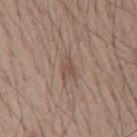Q: Is there a histopathology result?
A: catalogued during a skin exam; not biopsied
Q: Illumination type?
A: white-light
Q: Where on the body is the lesion?
A: the mid back
Q: What is the imaging modality?
A: ~15 mm tile from a whole-body skin photo
Q: What are the patient's age and sex?
A: male, approximately 40 years of age
Q: Lesion size?
A: ≈2.5 mm
Q: What did automated image analysis measure?
A: a border-irregularity index near 5.5/10, a color-variation rating of about 1/10, and a peripheral color-asymmetry measure near 0.5; a nevus-likeness score of about 0/100